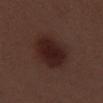Impression: Part of a total-body skin-imaging series; this lesion was reviewed on a skin check and was not flagged for biopsy. Clinical summary: A male patient roughly 70 years of age. A close-up tile cropped from a whole-body skin photograph, about 15 mm across. The recorded lesion diameter is about 5.5 mm. The lesion-visualizer software estimated a lesion area of about 21 mm², an eccentricity of roughly 0.45, and two-axis asymmetry of about 0.1. The software also gave a mean CIELAB color near L≈22 a*≈18 b*≈18 and a lesion-to-skin contrast of about 9.5 (normalized; higher = more distinct). The lesion is located on the right thigh. The tile uses white-light illumination.